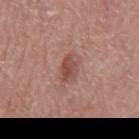Part of a total-body skin-imaging series; this lesion was reviewed on a skin check and was not flagged for biopsy. Cropped from a whole-body photographic skin survey; the tile spans about 15 mm. Imaged with white-light lighting. On the mid back. A male subject aged 68–72.